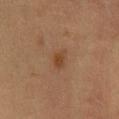Assessment:
No biopsy was performed on this lesion — it was imaged during a full skin examination and was not determined to be concerning.
Acquisition and patient details:
Cropped from a total-body skin-imaging series; the visible field is about 15 mm. The total-body-photography lesion software estimated an average lesion color of about L≈34 a*≈17 b*≈27 (CIELAB), about 7 CIELAB-L* units darker than the surrounding skin, and a normalized border contrast of about 7. And it measured a nevus-likeness score of about 40/100 and lesion-presence confidence of about 100/100. The subject is a female about 40 years old. On the chest. This is a cross-polarized tile.